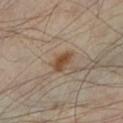Captured during whole-body skin photography for melanoma surveillance; the lesion was not biopsied.
Located on the left lower leg.
A 15 mm crop from a total-body photograph taken for skin-cancer surveillance.
A male patient, in their mid- to late 50s.